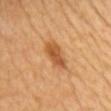No biopsy was performed on this lesion — it was imaged during a full skin examination and was not determined to be concerning.
The patient is a female in their mid- to late 40s.
The tile uses cross-polarized illumination.
The lesion-visualizer software estimated a lesion area of about 6.5 mm², an outline eccentricity of about 0.9 (0 = round, 1 = elongated), and a symmetry-axis asymmetry near 0.25. The analysis additionally found a border-irregularity rating of about 3/10 and peripheral color asymmetry of about 1.
Longest diameter approximately 4.5 mm.
A 15 mm crop from a total-body photograph taken for skin-cancer surveillance.
The lesion is on the chest.